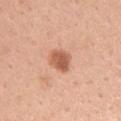Recorded during total-body skin imaging; not selected for excision or biopsy. Captured under white-light illumination. The lesion is located on the left upper arm. This image is a 15 mm lesion crop taken from a total-body photograph. About 3 mm across. A female subject about 30 years old.